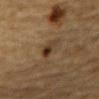Assessment:
The lesion was photographed on a routine skin check and not biopsied; there is no pathology result.
Background:
The lesion is located on the chest. A male subject approximately 85 years of age or older. Captured under cross-polarized illumination. A 15 mm close-up tile from a total-body photography series done for melanoma screening. The lesion's longest dimension is about 2.5 mm. An algorithmic analysis of the crop reported an area of roughly 3.5 mm². And it measured a border-irregularity index near 2.5/10, a within-lesion color-variation index near 7/10, and radial color variation of about 2.5.